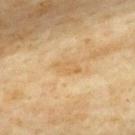| field | value |
|---|---|
| notes | imaged on a skin check; not biopsied |